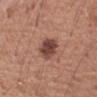biopsy status — total-body-photography surveillance lesion; no biopsy | acquisition — ~15 mm tile from a whole-body skin photo | lesion diameter — ~3.5 mm (longest diameter) | body site — the arm | tile lighting — white-light illumination | patient — female, aged approximately 55.